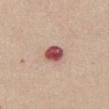| feature | finding |
|---|---|
| workup | total-body-photography surveillance lesion; no biopsy |
| subject | female, in their 40s |
| image-analysis metrics | a lesion–skin lightness drop of about 19 and a lesion-to-skin contrast of about 12.5 (normalized; higher = more distinct); an automated nevus-likeness rating near 0 out of 100 and lesion-presence confidence of about 100/100 |
| lighting | white-light |
| anatomic site | the abdomen |
| image source | total-body-photography crop, ~15 mm field of view |
| diameter | about 2.5 mm |